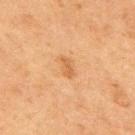A roughly 15 mm field-of-view crop from a total-body skin photograph. About 2.5 mm across. This is a cross-polarized tile. The lesion is located on the back. The patient is a male roughly 75 years of age.